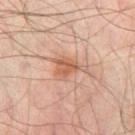Case summary:
– biopsy status · total-body-photography surveillance lesion; no biopsy
– anatomic site · the right thigh
– image · total-body-photography crop, ~15 mm field of view
– patient · male, in their mid-60s
– illumination · cross-polarized
– automated lesion analysis · a mean CIELAB color near L≈57 a*≈22 b*≈31 and a lesion–skin lightness drop of about 9; a within-lesion color-variation index near 4/10 and a peripheral color-asymmetry measure near 1.5; a classifier nevus-likeness of about 45/100 and a lesion-detection confidence of about 100/100